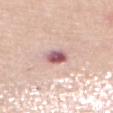{
  "biopsy_status": "not biopsied; imaged during a skin examination",
  "image": {
    "source": "total-body photography crop",
    "field_of_view_mm": 15
  },
  "lighting": "white-light",
  "automated_metrics": {
    "cielab_L": 58,
    "cielab_a": 26,
    "cielab_b": 18,
    "nevus_likeness_0_100": 0,
    "lesion_detection_confidence_0_100": 100
  },
  "patient": {
    "sex": "female",
    "age_approx": 70
  },
  "lesion_size": {
    "long_diameter_mm_approx": 3.0
  },
  "site": "mid back"
}